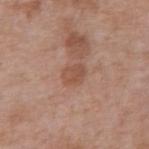– notes — catalogued during a skin exam; not biopsied
– image source — ~15 mm crop, total-body skin-cancer survey
– anatomic site — the abdomen
– subject — male, roughly 70 years of age
– lesion size — about 2.5 mm
– tile lighting — white-light